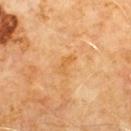follow-up: imaged on a skin check; not biopsied
subject: male, roughly 60 years of age
image source: ~15 mm crop, total-body skin-cancer survey
automated metrics: an area of roughly 3 mm², an eccentricity of roughly 0.85, and a shape-asymmetry score of about 0.45 (0 = symmetric); a mean CIELAB color near L≈61 a*≈23 b*≈46 and a normalized lesion–skin contrast near 5.5; a nevus-likeness score of about 0/100
body site: the chest
lesion size: ~2.5 mm (longest diameter)
tile lighting: cross-polarized illumination